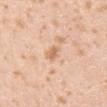| feature | finding |
|---|---|
| biopsy status | catalogued during a skin exam; not biopsied |
| body site | the arm |
| patient | male, approximately 25 years of age |
| acquisition | 15 mm crop, total-body photography |